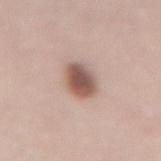Case summary:
• notes: no biopsy performed (imaged during a skin exam)
• image: ~15 mm tile from a whole-body skin photo
• tile lighting: white-light illumination
• subject: female, roughly 65 years of age
• site: the lower back
• lesion size: ≈3.5 mm